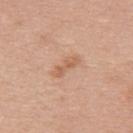follow-up: no biopsy performed (imaged during a skin exam)
automated lesion analysis: an area of roughly 4.5 mm², an eccentricity of roughly 0.9, and a symmetry-axis asymmetry near 0.35; a normalized border contrast of about 6
patient: female, aged 38 to 42
diameter: about 3.5 mm
image: ~15 mm tile from a whole-body skin photo
body site: the upper back
lighting: white-light illumination